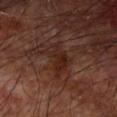{"biopsy_status": "not biopsied; imaged during a skin examination", "lighting": "cross-polarized", "image": {"source": "total-body photography crop", "field_of_view_mm": 15}, "lesion_size": {"long_diameter_mm_approx": 5.0}, "site": "left upper arm", "patient": {"sex": "male", "age_approx": 60}}